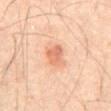Context: A male subject, aged approximately 65. A lesion tile, about 15 mm wide, cut from a 3D total-body photograph. An algorithmic analysis of the crop reported a shape eccentricity near 0.55 and a symmetry-axis asymmetry near 0.25. And it measured internal color variation of about 2.5 on a 0–10 scale. Captured under cross-polarized illumination. The recorded lesion diameter is about 3 mm. From the abdomen.